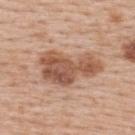Captured during whole-body skin photography for melanoma surveillance; the lesion was not biopsied. The lesion is on the upper back. The recorded lesion diameter is about 7 mm. The patient is a female aged approximately 65. A close-up tile cropped from a whole-body skin photograph, about 15 mm across. An algorithmic analysis of the crop reported a footprint of about 19 mm², an eccentricity of roughly 0.85, and two-axis asymmetry of about 0.4. It also reported a mean CIELAB color near L≈54 a*≈22 b*≈31. It also reported border irregularity of about 5 on a 0–10 scale, internal color variation of about 5 on a 0–10 scale, and radial color variation of about 1.5. The software also gave a lesion-detection confidence of about 100/100.